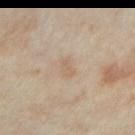No biopsy was performed on this lesion — it was imaged during a full skin examination and was not determined to be concerning. The recorded lesion diameter is about 2.5 mm. Located on the right forearm. A 15 mm crop from a total-body photograph taken for skin-cancer surveillance. Imaged with cross-polarized lighting. The patient is a female about 35 years old.